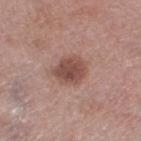| feature | finding |
|---|---|
| subject | female, aged approximately 70 |
| imaging modality | ~15 mm tile from a whole-body skin photo |
| site | the left thigh |
| tile lighting | white-light |